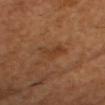notes: total-body-photography surveillance lesion; no biopsy
image: 15 mm crop, total-body photography
illumination: cross-polarized
patient: male, aged 43 to 47
lesion diameter: ≈3.5 mm
location: the head or neck
automated metrics: a shape eccentricity near 0.8 and a symmetry-axis asymmetry near 0.45; a lesion color around L≈38 a*≈21 b*≈32 in CIELAB, a lesion–skin lightness drop of about 6, and a normalized border contrast of about 5.5